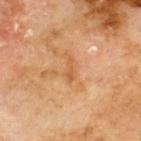Part of a total-body skin-imaging series; this lesion was reviewed on a skin check and was not flagged for biopsy.
The lesion is on the upper back.
A male subject, about 70 years old.
A lesion tile, about 15 mm wide, cut from a 3D total-body photograph.
Measured at roughly 3 mm in maximum diameter.